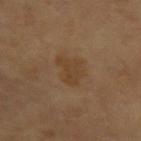<case>
<biopsy_status>not biopsied; imaged during a skin examination</biopsy_status>
<lighting>cross-polarized</lighting>
<site>left forearm</site>
<patient>
  <sex>male</sex>
  <age_approx>65</age_approx>
</patient>
<lesion_size>
  <long_diameter_mm_approx>3.5</long_diameter_mm_approx>
</lesion_size>
<image>
  <source>total-body photography crop</source>
  <field_of_view_mm>15</field_of_view_mm>
</image>
</case>